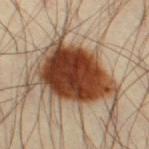<record>
<biopsy_status>not biopsied; imaged during a skin examination</biopsy_status>
<site>right thigh</site>
<automated_metrics>
  <cielab_L>41</cielab_L>
  <cielab_a>22</cielab_a>
  <cielab_b>32</cielab_b>
  <vs_skin_darker_L>22.0</vs_skin_darker_L>
  <vs_skin_contrast_norm>16.5</vs_skin_contrast_norm>
  <border_irregularity_0_10>3.5</border_irregularity_0_10>
  <color_variation_0_10>7.5</color_variation_0_10>
</automated_metrics>
<image>
  <source>total-body photography crop</source>
  <field_of_view_mm>15</field_of_view_mm>
</image>
<patient>
  <sex>male</sex>
  <age_approx>40</age_approx>
</patient>
<lighting>cross-polarized</lighting>
<lesion_size>
  <long_diameter_mm_approx>9.0</long_diameter_mm_approx>
</lesion_size>
</record>